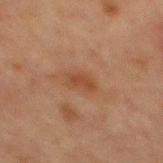This lesion was catalogued during total-body skin photography and was not selected for biopsy. The recorded lesion diameter is about 2.5 mm. The lesion is located on the abdomen. A male subject, aged around 65. A lesion tile, about 15 mm wide, cut from a 3D total-body photograph. Captured under cross-polarized illumination. The total-body-photography lesion software estimated border irregularity of about 3.5 on a 0–10 scale and a peripheral color-asymmetry measure near 0.5. And it measured a classifier nevus-likeness of about 15/100 and a detector confidence of about 100 out of 100 that the crop contains a lesion.